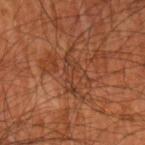biopsy status=catalogued during a skin exam; not biopsied
diameter=about 5 mm
anatomic site=the arm
patient=male, aged 43–47
lighting=cross-polarized
image source=~15 mm tile from a whole-body skin photo